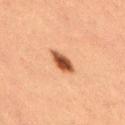Q: Was this lesion biopsied?
A: total-body-photography surveillance lesion; no biopsy
Q: Where on the body is the lesion?
A: the right thigh
Q: How was this image acquired?
A: ~15 mm tile from a whole-body skin photo
Q: How large is the lesion?
A: about 3.5 mm
Q: Patient demographics?
A: female, aged 38–42
Q: What lighting was used for the tile?
A: cross-polarized